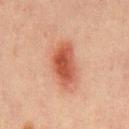Q: Was this lesion biopsied?
A: total-body-photography surveillance lesion; no biopsy